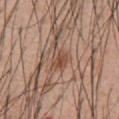Impression: Recorded during total-body skin imaging; not selected for excision or biopsy. Image and clinical context: A male patient, approximately 55 years of age. A close-up tile cropped from a whole-body skin photograph, about 15 mm across. From the front of the torso. Captured under white-light illumination.